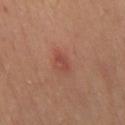Part of a total-body skin-imaging series; this lesion was reviewed on a skin check and was not flagged for biopsy.
Automated tile analysis of the lesion measured a lesion area of about 3 mm², a shape eccentricity near 0.8, and two-axis asymmetry of about 0.35. It also reported a mean CIELAB color near L≈46 a*≈27 b*≈27 and a lesion–skin lightness drop of about 7.
This image is a 15 mm lesion crop taken from a total-body photograph.
On the right thigh.
This is a cross-polarized tile.
Measured at roughly 2.5 mm in maximum diameter.
A female subject, aged around 40.